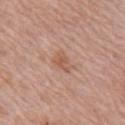Q: Was a biopsy performed?
A: catalogued during a skin exam; not biopsied
Q: What is the lesion's diameter?
A: about 3 mm
Q: How was the tile lit?
A: white-light illumination
Q: Lesion location?
A: the right upper arm
Q: What are the patient's age and sex?
A: female, approximately 60 years of age
Q: How was this image acquired?
A: 15 mm crop, total-body photography